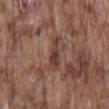follow-up — imaged on a skin check; not biopsied
tile lighting — white-light
location — the lower back
imaging modality — ~15 mm tile from a whole-body skin photo
subject — male, aged approximately 75
image-analysis metrics — a lesion area of about 5 mm², a shape eccentricity near 0.9, and a symmetry-axis asymmetry near 0.4; a within-lesion color-variation index near 2/10 and radial color variation of about 0.5
diameter — about 4 mm